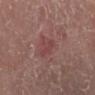Assessment: The lesion was photographed on a routine skin check and not biopsied; there is no pathology result. Clinical summary: The patient is a female aged around 80. The recorded lesion diameter is about 3 mm. On the right leg. A lesion tile, about 15 mm wide, cut from a 3D total-body photograph.